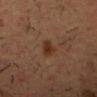<lesion>
<biopsy_status>not biopsied; imaged during a skin examination</biopsy_status>
<lighting>cross-polarized</lighting>
<patient>
  <sex>male</sex>
  <age_approx>35</age_approx>
</patient>
<image>
  <source>total-body photography crop</source>
  <field_of_view_mm>15</field_of_view_mm>
</image>
<site>head or neck</site>
</lesion>